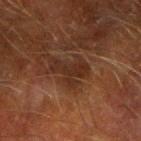notes — imaged on a skin check; not biopsied.